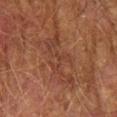{"biopsy_status": "not biopsied; imaged during a skin examination", "lesion_size": {"long_diameter_mm_approx": 7.0}, "site": "left forearm", "patient": {"sex": "male", "age_approx": 75}, "image": {"source": "total-body photography crop", "field_of_view_mm": 15}, "automated_metrics": {"cielab_L": 32, "cielab_a": 19, "cielab_b": 25, "vs_skin_darker_L": 5.0, "nevus_likeness_0_100": 0, "lesion_detection_confidence_0_100": 60}}